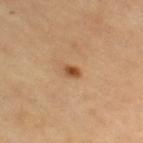{
  "biopsy_status": "not biopsied; imaged during a skin examination",
  "site": "right thigh",
  "patient": {
    "sex": "female",
    "age_approx": 70
  },
  "lesion_size": {
    "long_diameter_mm_approx": 2.0
  },
  "lighting": "cross-polarized",
  "image": {
    "source": "total-body photography crop",
    "field_of_view_mm": 15
  }
}